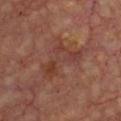Q: Was a biopsy performed?
A: total-body-photography surveillance lesion; no biopsy
Q: What is the anatomic site?
A: the chest
Q: What are the patient's age and sex?
A: about 65 years old
Q: What did automated image analysis measure?
A: a lesion color around L≈38 a*≈23 b*≈25 in CIELAB and roughly 6 lightness units darker than nearby skin; a within-lesion color-variation index near 6/10
Q: What kind of image is this?
A: total-body-photography crop, ~15 mm field of view
Q: How was the tile lit?
A: cross-polarized illumination